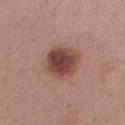biopsy_status: not biopsied; imaged during a skin examination